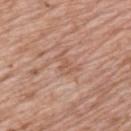Q: Was this lesion biopsied?
A: total-body-photography surveillance lesion; no biopsy
Q: What is the imaging modality?
A: ~15 mm crop, total-body skin-cancer survey
Q: How was the tile lit?
A: white-light illumination
Q: What is the anatomic site?
A: the upper back
Q: Patient demographics?
A: male, aged 58–62
Q: How large is the lesion?
A: ≈3 mm